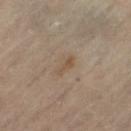biopsy_status: not biopsied; imaged during a skin examination
patient:
  sex: female
  age_approx: 60
automated_metrics:
  border_irregularity_0_10: 3.5
  color_variation_0_10: 0.5
  peripheral_color_asymmetry: 0.0
  nevus_likeness_0_100: 5
  lesion_detection_confidence_0_100: 100
image:
  source: total-body photography crop
  field_of_view_mm: 15
lighting: cross-polarized
site: leg